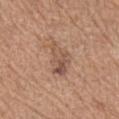The lesion was tiled from a total-body skin photograph and was not biopsied. A region of skin cropped from a whole-body photographic capture, roughly 15 mm wide. The total-body-photography lesion software estimated a color-variation rating of about 6/10. The software also gave an automated nevus-likeness rating near 15 out of 100. The patient is a female about 55 years old. The recorded lesion diameter is about 4.5 mm. Imaged with white-light lighting. Located on the left forearm.